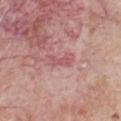Findings:
• workup: catalogued during a skin exam; not biopsied
• acquisition: ~15 mm tile from a whole-body skin photo
• body site: the front of the torso
• TBP lesion metrics: a footprint of about 4 mm² and a shape eccentricity near 0.9; a border-irregularity index near 4.5/10 and peripheral color asymmetry of about 0.5
• lighting: white-light illumination
• diameter: ≈3.5 mm
• patient: male, approximately 65 years of age